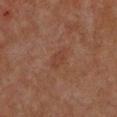Impression:
This lesion was catalogued during total-body skin photography and was not selected for biopsy.
Context:
Imaged with cross-polarized lighting. A female patient roughly 70 years of age. Cropped from a whole-body photographic skin survey; the tile spans about 15 mm. The lesion is on the chest. About 3 mm across.